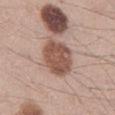The lesion was photographed on a routine skin check and not biopsied; there is no pathology result.
A lesion tile, about 15 mm wide, cut from a 3D total-body photograph.
Located on the mid back.
The subject is a male in their mid-30s.
Imaged with white-light lighting.
The total-body-photography lesion software estimated a mean CIELAB color near L≈52 a*≈20 b*≈26. And it measured a color-variation rating of about 3/10 and peripheral color asymmetry of about 1.